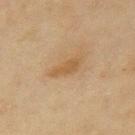Imaged during a routine full-body skin examination; the lesion was not biopsied and no histopathology is available. From the left upper arm. The subject is a male aged approximately 45. Longest diameter approximately 3.5 mm. The tile uses cross-polarized illumination. Cropped from a total-body skin-imaging series; the visible field is about 15 mm.